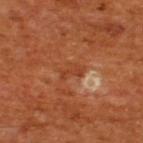{"biopsy_status": "not biopsied; imaged during a skin examination", "automated_metrics": {"eccentricity": 0.85, "shape_asymmetry": 0.4, "cielab_L": 40, "cielab_a": 28, "cielab_b": 35, "vs_skin_darker_L": 6.0, "vs_skin_contrast_norm": 5.5, "border_irregularity_0_10": 4.5, "color_variation_0_10": 1.0, "peripheral_color_asymmetry": 0.0, "nevus_likeness_0_100": 0, "lesion_detection_confidence_0_100": 70}, "lesion_size": {"long_diameter_mm_approx": 3.0}, "site": "upper back", "image": {"source": "total-body photography crop", "field_of_view_mm": 15}, "lighting": "cross-polarized", "patient": {"sex": "male", "age_approx": 65}}